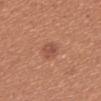<tbp_lesion>
  <biopsy_status>not biopsied; imaged during a skin examination</biopsy_status>
  <image>
    <source>total-body photography crop</source>
    <field_of_view_mm>15</field_of_view_mm>
  </image>
  <patient>
    <sex>female</sex>
    <age_approx>50</age_approx>
  </patient>
  <lighting>white-light</lighting>
  <lesion_size>
    <long_diameter_mm_approx>3.0</long_diameter_mm_approx>
  </lesion_size>
  <site>upper back</site>
</tbp_lesion>